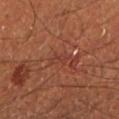Impression: No biopsy was performed on this lesion — it was imaged during a full skin examination and was not determined to be concerning. Image and clinical context: Approximately 2.5 mm at its widest. On the left lower leg. The patient is a male aged 33–37. A 15 mm crop from a total-body photograph taken for skin-cancer surveillance. This is a cross-polarized tile.